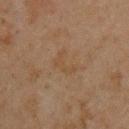Located on the chest.
Measured at roughly 3 mm in maximum diameter.
Imaged with cross-polarized lighting.
A 15 mm close-up tile from a total-body photography series done for melanoma screening.
A male subject in their mid- to late 40s.